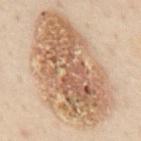Case summary:
• notes · total-body-photography surveillance lesion; no biopsy
• subject · male, aged 48 to 52
• image · ~15 mm tile from a whole-body skin photo
• site · the mid back
• image-analysis metrics · an average lesion color of about L≈51 a*≈13 b*≈27 (CIELAB) and a normalized border contrast of about 8
• illumination · cross-polarized
• size · ~13.5 mm (longest diameter)